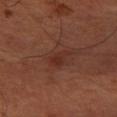The lesion was tiled from a total-body skin photograph and was not biopsied.
Approximately 2.5 mm at its widest.
A region of skin cropped from a whole-body photographic capture, roughly 15 mm wide.
A male patient aged around 60.
Captured under cross-polarized illumination.
The lesion is located on the right forearm.